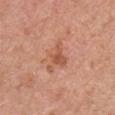Assessment:
Part of a total-body skin-imaging series; this lesion was reviewed on a skin check and was not flagged for biopsy.
Context:
Approximately 4 mm at its widest. This is a white-light tile. The total-body-photography lesion software estimated a within-lesion color-variation index near 3/10 and a peripheral color-asymmetry measure near 1. From the left upper arm. A roughly 15 mm field-of-view crop from a total-body skin photograph. A male patient aged 68 to 72.